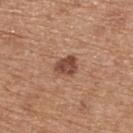| field | value |
|---|---|
| workup | total-body-photography surveillance lesion; no biopsy |
| location | the upper back |
| patient | female, in their mid- to late 50s |
| image source | ~15 mm tile from a whole-body skin photo |